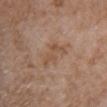The lesion was photographed on a routine skin check and not biopsied; there is no pathology result.
A 15 mm crop from a total-body photograph taken for skin-cancer surveillance.
This is a white-light tile.
A female subject, aged approximately 85 or older.
The lesion is located on the chest.